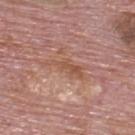Clinical impression:
Imaged during a routine full-body skin examination; the lesion was not biopsied and no histopathology is available.
Clinical summary:
Measured at roughly 4.5 mm in maximum diameter. An algorithmic analysis of the crop reported a footprint of about 5 mm², an outline eccentricity of about 0.95 (0 = round, 1 = elongated), and two-axis asymmetry of about 0.5. The tile uses white-light illumination. A male patient about 75 years old. From the upper back. A region of skin cropped from a whole-body photographic capture, roughly 15 mm wide.